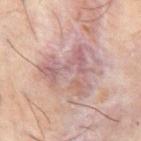This lesion was catalogued during total-body skin photography and was not selected for biopsy. A male subject about 60 years old. The lesion is on the abdomen. A 15 mm crop from a total-body photograph taken for skin-cancer surveillance.